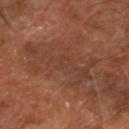biopsy status: imaged on a skin check; not biopsied, site: the right lower leg, patient: in their mid- to late 60s, acquisition: ~15 mm tile from a whole-body skin photo.